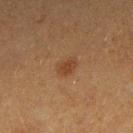Clinical impression:
This lesion was catalogued during total-body skin photography and was not selected for biopsy.
Clinical summary:
Cropped from a whole-body photographic skin survey; the tile spans about 15 mm. A female subject aged approximately 40. The lesion is on the right forearm.